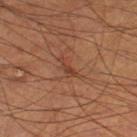{
  "biopsy_status": "not biopsied; imaged during a skin examination",
  "site": "leg",
  "image": {
    "source": "total-body photography crop",
    "field_of_view_mm": 15
  },
  "automated_metrics": {
    "border_irregularity_0_10": 5.5,
    "peripheral_color_asymmetry": 0.0,
    "nevus_likeness_0_100": 0
  },
  "patient": {
    "sex": "male",
    "age_approx": 70
  }
}